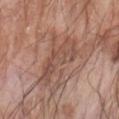Assessment:
The lesion was photographed on a routine skin check and not biopsied; there is no pathology result.
Acquisition and patient details:
The lesion is on the left forearm. A male patient, about 60 years old. A roughly 15 mm field-of-view crop from a total-body skin photograph. Automated tile analysis of the lesion measured a lesion area of about 12 mm², an outline eccentricity of about 0.9 (0 = round, 1 = elongated), and a shape-asymmetry score of about 0.4 (0 = symmetric). The software also gave a lesion color around L≈50 a*≈20 b*≈26 in CIELAB. The analysis additionally found a border-irregularity index near 6.5/10, a color-variation rating of about 4/10, and radial color variation of about 1.5. The software also gave a classifier nevus-likeness of about 0/100 and a lesion-detection confidence of about 95/100. The tile uses white-light illumination.